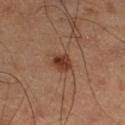Imaged during a routine full-body skin examination; the lesion was not biopsied and no histopathology is available.
Measured at roughly 2.5 mm in maximum diameter.
Captured under cross-polarized illumination.
Located on the left lower leg.
The subject is a male aged approximately 60.
A roughly 15 mm field-of-view crop from a total-body skin photograph.